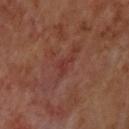Notes:
* biopsy status: no biopsy performed (imaged during a skin exam)
* anatomic site: the back
* patient: male, roughly 70 years of age
* tile lighting: cross-polarized
* image-analysis metrics: an outline eccentricity of about 0.8 (0 = round, 1 = elongated) and a symmetry-axis asymmetry near 0.6; about 5 CIELAB-L* units darker than the surrounding skin and a normalized border contrast of about 5; a classifier nevus-likeness of about 0/100 and lesion-presence confidence of about 65/100
* imaging modality: ~15 mm tile from a whole-body skin photo